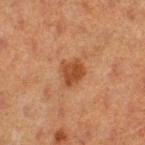Impression: Imaged during a routine full-body skin examination; the lesion was not biopsied and no histopathology is available. Clinical summary: The recorded lesion diameter is about 3 mm. A female patient aged 38–42. The lesion is on the left thigh. The tile uses cross-polarized illumination. A close-up tile cropped from a whole-body skin photograph, about 15 mm across. Automated tile analysis of the lesion measured a footprint of about 6.5 mm², a shape eccentricity near 0.5, and two-axis asymmetry of about 0.25. The analysis additionally found a classifier nevus-likeness of about 70/100.